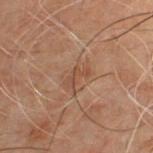Q: Was this lesion biopsied?
A: catalogued during a skin exam; not biopsied
Q: Where on the body is the lesion?
A: the front of the torso
Q: Who is the patient?
A: male, roughly 50 years of age
Q: How was the tile lit?
A: cross-polarized
Q: What is the imaging modality?
A: ~15 mm tile from a whole-body skin photo
Q: Lesion size?
A: about 3 mm